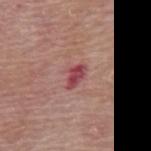Impression:
Part of a total-body skin-imaging series; this lesion was reviewed on a skin check and was not flagged for biopsy.
Acquisition and patient details:
A male patient, about 80 years old. The lesion is on the mid back. This is a white-light tile. A 15 mm crop from a total-body photograph taken for skin-cancer surveillance.